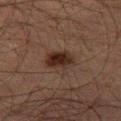Impression: This lesion was catalogued during total-body skin photography and was not selected for biopsy. Acquisition and patient details: Automated image analysis of the tile measured a color-variation rating of about 4/10 and radial color variation of about 1. The analysis additionally found a lesion-detection confidence of about 100/100. Approximately 3.5 mm at its widest. A lesion tile, about 15 mm wide, cut from a 3D total-body photograph. The tile uses cross-polarized illumination. On the leg. A male subject, roughly 50 years of age.